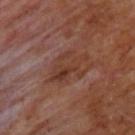The lesion was tiled from a total-body skin photograph and was not biopsied. A lesion tile, about 15 mm wide, cut from a 3D total-body photograph. Captured under cross-polarized illumination. The lesion is on the chest. A male subject, aged 58 to 62. About 5.5 mm across. The total-body-photography lesion software estimated an area of roughly 11 mm², a shape eccentricity near 0.8, and two-axis asymmetry of about 0.35. The software also gave a border-irregularity index near 6/10, a within-lesion color-variation index near 6/10, and peripheral color asymmetry of about 2. And it measured a classifier nevus-likeness of about 0/100 and a detector confidence of about 95 out of 100 that the crop contains a lesion.